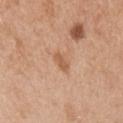Part of a total-body skin-imaging series; this lesion was reviewed on a skin check and was not flagged for biopsy.